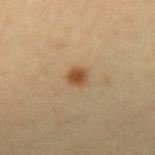Assessment:
Recorded during total-body skin imaging; not selected for excision or biopsy.
Clinical summary:
About 2.5 mm across. This image is a 15 mm lesion crop taken from a total-body photograph. A female subject, aged 28 to 32. Automated image analysis of the tile measured a footprint of about 4 mm² and a shape-asymmetry score of about 0.15 (0 = symmetric). It also reported a lesion color around L≈46 a*≈15 b*≈33 in CIELAB. The software also gave a nevus-likeness score of about 100/100. On the right forearm.